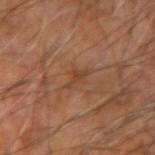Q: Was a biopsy performed?
A: total-body-photography surveillance lesion; no biopsy
Q: What are the patient's age and sex?
A: in their mid-60s
Q: What is the imaging modality?
A: ~15 mm crop, total-body skin-cancer survey
Q: What is the anatomic site?
A: the arm